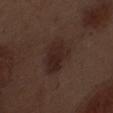Captured under white-light illumination. The lesion's longest dimension is about 5 mm. A male patient, in their 70s. The lesion is located on the front of the torso. A 15 mm crop from a total-body photograph taken for skin-cancer surveillance.